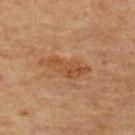Q: How was the tile lit?
A: cross-polarized
Q: Automated lesion metrics?
A: a mean CIELAB color near L≈44 a*≈21 b*≈34 and a normalized border contrast of about 7; a border-irregularity rating of about 5.5/10 and peripheral color asymmetry of about 1
Q: What is the anatomic site?
A: the upper back
Q: What are the patient's age and sex?
A: male, in their mid- to late 60s
Q: How was this image acquired?
A: total-body-photography crop, ~15 mm field of view